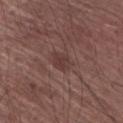Case summary:
– subject · male, roughly 65 years of age
– anatomic site · the right upper arm
– size · ≈3 mm
– image · total-body-photography crop, ~15 mm field of view